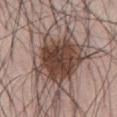workup — imaged on a skin check; not biopsied
image source — total-body-photography crop, ~15 mm field of view
subject — male, aged around 70
anatomic site — the abdomen